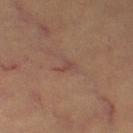Assessment:
This lesion was catalogued during total-body skin photography and was not selected for biopsy.
Clinical summary:
A lesion tile, about 15 mm wide, cut from a 3D total-body photograph. From the right thigh. This is a cross-polarized tile. A female patient, approximately 30 years of age. Automated image analysis of the tile measured a mean CIELAB color near L≈42 a*≈20 b*≈23, a lesion–skin lightness drop of about 6, and a normalized border contrast of about 5. And it measured a border-irregularity index near 5.5/10, internal color variation of about 0 on a 0–10 scale, and peripheral color asymmetry of about 0.